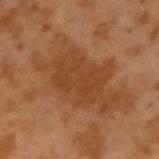Notes:
• biopsy status — no biopsy performed (imaged during a skin exam)
• subject — male, in their mid- to late 40s
• body site — the right upper arm
• imaging modality — 15 mm crop, total-body photography